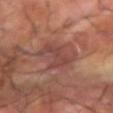This lesion was catalogued during total-body skin photography and was not selected for biopsy. The lesion's longest dimension is about 5 mm. Imaged with cross-polarized lighting. An algorithmic analysis of the crop reported a mean CIELAB color near L≈42 a*≈23 b*≈23, about 7 CIELAB-L* units darker than the surrounding skin, and a normalized lesion–skin contrast near 6. The analysis additionally found internal color variation of about 2 on a 0–10 scale and a peripheral color-asymmetry measure near 0.5. And it measured a classifier nevus-likeness of about 0/100 and a lesion-detection confidence of about 95/100. The patient is a male aged approximately 60. A lesion tile, about 15 mm wide, cut from a 3D total-body photograph. The lesion is located on the right forearm.